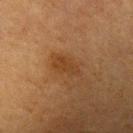Clinical impression:
Captured during whole-body skin photography for melanoma surveillance; the lesion was not biopsied.
Image and clinical context:
Located on the left upper arm. A female subject, about 55 years old. Automated tile analysis of the lesion measured a lesion area of about 4.5 mm², a shape eccentricity near 0.85, and a shape-asymmetry score of about 0.3 (0 = symmetric). The software also gave an average lesion color of about L≈33 a*≈18 b*≈31 (CIELAB), roughly 6 lightness units darker than nearby skin, and a normalized lesion–skin contrast near 6.5. The analysis additionally found a within-lesion color-variation index near 2/10 and a peripheral color-asymmetry measure near 0.5. A roughly 15 mm field-of-view crop from a total-body skin photograph. The tile uses cross-polarized illumination.